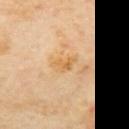<case>
<biopsy_status>not biopsied; imaged during a skin examination</biopsy_status>
<image>
  <source>total-body photography crop</source>
  <field_of_view_mm>15</field_of_view_mm>
</image>
<automated_metrics>
  <area_mm2_approx>4.5</area_mm2_approx>
  <shape_asymmetry>0.35</shape_asymmetry>
  <cielab_L>67</cielab_L>
  <cielab_a>19</cielab_a>
  <cielab_b>44</cielab_b>
  <vs_skin_darker_L>7.0</vs_skin_darker_L>
  <border_irregularity_0_10>3.5</border_irregularity_0_10>
  <color_variation_0_10>3.0</color_variation_0_10>
  <peripheral_color_asymmetry>1.0</peripheral_color_asymmetry>
  <nevus_likeness_0_100>0</nevus_likeness_0_100>
  <lesion_detection_confidence_0_100>100</lesion_detection_confidence_0_100>
</automated_metrics>
<site>mid back</site>
<lesion_size>
  <long_diameter_mm_approx>3.0</long_diameter_mm_approx>
</lesion_size>
<patient>
  <sex>male</sex>
  <age_approx>65</age_approx>
</patient>
</case>